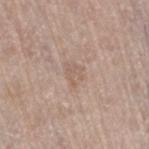biopsy status — imaged on a skin check; not biopsied
size — about 2.5 mm
imaging modality — ~15 mm tile from a whole-body skin photo
subject — female, in their 60s
TBP lesion metrics — a footprint of about 3.5 mm², an outline eccentricity of about 0.7 (0 = round, 1 = elongated), and a shape-asymmetry score of about 0.35 (0 = symmetric); an average lesion color of about L≈59 a*≈16 b*≈26 (CIELAB), a lesion–skin lightness drop of about 6, and a normalized lesion–skin contrast near 4.5; a border-irregularity index near 3.5/10, a color-variation rating of about 1.5/10, and peripheral color asymmetry of about 0.5
tile lighting — white-light
location — the right thigh